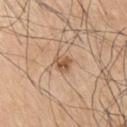No biopsy was performed on this lesion — it was imaged during a full skin examination and was not determined to be concerning.
Captured under white-light illumination.
A lesion tile, about 15 mm wide, cut from a 3D total-body photograph.
A male patient, about 75 years old.
On the right upper arm.